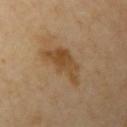workup: no biopsy performed (imaged during a skin exam)
location: the left upper arm
lesion size: about 6 mm
tile lighting: cross-polarized illumination
imaging modality: ~15 mm tile from a whole-body skin photo
patient: female, aged around 65